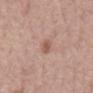workup: no biopsy performed (imaged during a skin exam); patient: male, aged around 60; image: ~15 mm tile from a whole-body skin photo; site: the mid back.